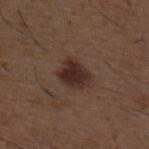No biopsy was performed on this lesion — it was imaged during a full skin examination and was not determined to be concerning.
A close-up tile cropped from a whole-body skin photograph, about 15 mm across.
The lesion's longest dimension is about 4 mm.
A male patient, aged approximately 50.
Imaged with white-light lighting.
From the mid back.